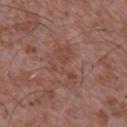biopsy status = imaged on a skin check; not biopsied | image source = 15 mm crop, total-body photography | automated metrics = a lesion–skin lightness drop of about 5 and a normalized border contrast of about 5; a within-lesion color-variation index near 3.5/10 and peripheral color asymmetry of about 1; a nevus-likeness score of about 0/100 and lesion-presence confidence of about 100/100 | patient = male, aged 43–47 | size = ~5 mm (longest diameter) | anatomic site = the chest.